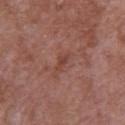Part of a total-body skin-imaging series; this lesion was reviewed on a skin check and was not flagged for biopsy. Imaged with white-light lighting. About 2.5 mm across. The lesion is located on the right upper arm. A close-up tile cropped from a whole-body skin photograph, about 15 mm across. An algorithmic analysis of the crop reported a shape eccentricity near 0.9 and two-axis asymmetry of about 0.35. The software also gave a mean CIELAB color near L≈43 a*≈24 b*≈27, a lesion–skin lightness drop of about 7, and a lesion-to-skin contrast of about 6 (normalized; higher = more distinct). And it measured internal color variation of about 0 on a 0–10 scale and peripheral color asymmetry of about 0. The patient is a female aged 68 to 72.